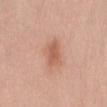Impression: The lesion was photographed on a routine skin check and not biopsied; there is no pathology result. Clinical summary: The total-body-photography lesion software estimated an automated nevus-likeness rating near 85 out of 100 and a detector confidence of about 100 out of 100 that the crop contains a lesion. A 15 mm close-up tile from a total-body photography series done for melanoma screening. This is a white-light tile. The subject is a female approximately 65 years of age. Measured at roughly 3.5 mm in maximum diameter. The lesion is located on the back.